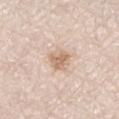workup — imaged on a skin check; not biopsied | patient — male, aged 78–82 | anatomic site — the right thigh | size — ≈3 mm | imaging modality — ~15 mm tile from a whole-body skin photo | illumination — white-light illumination | TBP lesion metrics — a mean CIELAB color near L≈68 a*≈16 b*≈30 and a normalized border contrast of about 6.5; a nevus-likeness score of about 20/100 and a lesion-detection confidence of about 100/100.